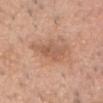Background:
A lesion tile, about 15 mm wide, cut from a 3D total-body photograph. Automated tile analysis of the lesion measured a footprint of about 8 mm², an eccentricity of roughly 0.8, and a shape-asymmetry score of about 0.35 (0 = symmetric). The analysis additionally found a classifier nevus-likeness of about 5/100. Imaged with white-light lighting. Approximately 4 mm at its widest. Located on the head or neck. A male subject, aged approximately 40.